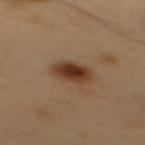Recorded during total-body skin imaging; not selected for excision or biopsy.
The subject is a male aged 53 to 57.
A 15 mm close-up tile from a total-body photography series done for melanoma screening.
Located on the mid back.
This is a cross-polarized tile.